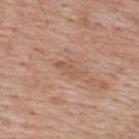Background: About 3.5 mm across. A roughly 15 mm field-of-view crop from a total-body skin photograph. From the back. A male patient, approximately 70 years of age.